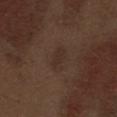biopsy status = total-body-photography surveillance lesion; no biopsy
anatomic site = the abdomen
tile lighting = white-light illumination
patient = male, aged approximately 70
imaging modality = total-body-photography crop, ~15 mm field of view